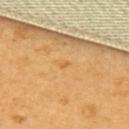Context:
Measured at roughly 1 mm in maximum diameter. A 15 mm close-up tile from a total-body photography series done for melanoma screening. Automated image analysis of the tile measured a lesion area of about 1 mm², an eccentricity of roughly 0.8, and a shape-asymmetry score of about 0.5 (0 = symmetric). And it measured a lesion color around L≈63 a*≈22 b*≈48 in CIELAB and roughly 7 lightness units darker than nearby skin. The analysis additionally found a border-irregularity rating of about 3.5/10 and a within-lesion color-variation index near 0/10. On the upper back. A female subject, aged 53–57.